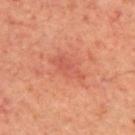The lesion was photographed on a routine skin check and not biopsied; there is no pathology result.
Captured under cross-polarized illumination.
The recorded lesion diameter is about 4 mm.
A male subject aged approximately 70.
The lesion is on the upper back.
Cropped from a total-body skin-imaging series; the visible field is about 15 mm.